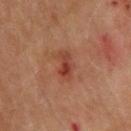Impression: The lesion was photographed on a routine skin check and not biopsied; there is no pathology result. Context: Captured under cross-polarized illumination. The patient is a male in their mid- to late 60s. A 15 mm close-up extracted from a 3D total-body photography capture. Measured at roughly 3 mm in maximum diameter. The lesion is on the back.